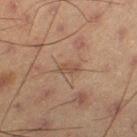Q: Is there a histopathology result?
A: total-body-photography surveillance lesion; no biopsy